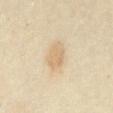Case summary:
- location · the abdomen
- imaging modality · ~15 mm tile from a whole-body skin photo
- patient · female, approximately 35 years of age